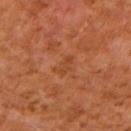Assessment: Recorded during total-body skin imaging; not selected for excision or biopsy. Image and clinical context: The lesion-visualizer software estimated a footprint of about 3 mm², an outline eccentricity of about 0.9 (0 = round, 1 = elongated), and a shape-asymmetry score of about 0.45 (0 = symmetric). The patient is a male aged 58 to 62. On the right upper arm. About 3 mm across. The tile uses cross-polarized illumination. A roughly 15 mm field-of-view crop from a total-body skin photograph.